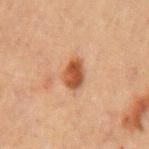The lesion was photographed on a routine skin check and not biopsied; there is no pathology result.
The lesion is located on the left upper arm.
A region of skin cropped from a whole-body photographic capture, roughly 15 mm wide.
The subject is a male roughly 50 years of age.